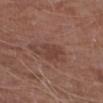{"biopsy_status": "not biopsied; imaged during a skin examination", "patient": {"sex": "male", "age_approx": 65}, "lighting": "white-light", "site": "right upper arm", "image": {"source": "total-body photography crop", "field_of_view_mm": 15}, "lesion_size": {"long_diameter_mm_approx": 3.0}}